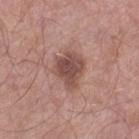Assessment: The lesion was photographed on a routine skin check and not biopsied; there is no pathology result. Context: From the right lower leg. Captured under white-light illumination. A male subject roughly 55 years of age. Longest diameter approximately 4 mm. A close-up tile cropped from a whole-body skin photograph, about 15 mm across. Automated image analysis of the tile measured an area of roughly 11 mm², a shape eccentricity near 0.6, and a shape-asymmetry score of about 0.25 (0 = symmetric). And it measured a classifier nevus-likeness of about 45/100 and lesion-presence confidence of about 100/100.